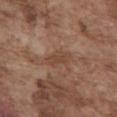biopsy status — imaged on a skin check; not biopsied